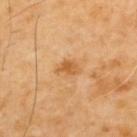A region of skin cropped from a whole-body photographic capture, roughly 15 mm wide.
The patient is a male approximately 70 years of age.
On the upper back.
Imaged with cross-polarized lighting.
The total-body-photography lesion software estimated a lesion area of about 4 mm², an eccentricity of roughly 0.75, and two-axis asymmetry of about 0.3. The software also gave a mean CIELAB color near L≈57 a*≈23 b*≈43, a lesion–skin lightness drop of about 9, and a normalized border contrast of about 7.
The lesion's longest dimension is about 2.5 mm.